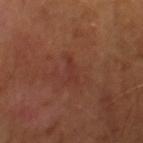Impression: Imaged during a routine full-body skin examination; the lesion was not biopsied and no histopathology is available. Clinical summary: A male patient, aged 63–67. Captured under cross-polarized illumination. This image is a 15 mm lesion crop taken from a total-body photograph. About 3.5 mm across.